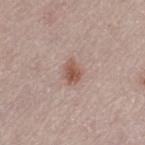The lesion was tiled from a total-body skin photograph and was not biopsied.
This is a white-light tile.
A female subject aged 48 to 52.
Measured at roughly 3 mm in maximum diameter.
On the left thigh.
A 15 mm crop from a total-body photograph taken for skin-cancer surveillance.